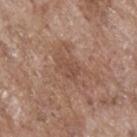Recorded during total-body skin imaging; not selected for excision or biopsy.
A lesion tile, about 15 mm wide, cut from a 3D total-body photograph.
A male subject about 70 years old.
On the upper back.
Approximately 3 mm at its widest.